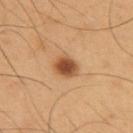{
  "biopsy_status": "not biopsied; imaged during a skin examination",
  "patient": {
    "sex": "male",
    "age_approx": 55
  },
  "site": "upper back",
  "lighting": "cross-polarized",
  "image": {
    "source": "total-body photography crop",
    "field_of_view_mm": 15
  }
}